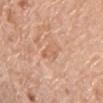No biopsy was performed on this lesion — it was imaged during a full skin examination and was not determined to be concerning. The lesion's longest dimension is about 4 mm. Located on the chest. The patient is a male aged 78–82. A 15 mm crop from a total-body photograph taken for skin-cancer surveillance.